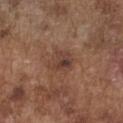Captured during whole-body skin photography for melanoma surveillance; the lesion was not biopsied. A 15 mm close-up tile from a total-body photography series done for melanoma screening. On the chest. The patient is a male about 75 years old.